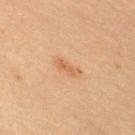Captured during whole-body skin photography for melanoma surveillance; the lesion was not biopsied. A male patient aged 58–62. Located on the upper back. An algorithmic analysis of the crop reported a lesion color around L≈50 a*≈20 b*≈33 in CIELAB, about 7 CIELAB-L* units darker than the surrounding skin, and a normalized lesion–skin contrast near 6. And it measured border irregularity of about 5 on a 0–10 scale and a within-lesion color-variation index near 0/10. The software also gave a nevus-likeness score of about 55/100 and a detector confidence of about 100 out of 100 that the crop contains a lesion. This is a cross-polarized tile. Measured at roughly 3 mm in maximum diameter. A roughly 15 mm field-of-view crop from a total-body skin photograph.